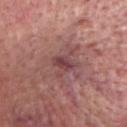<record>
<biopsy_status>not biopsied; imaged during a skin examination</biopsy_status>
<site>head or neck</site>
<lighting>white-light</lighting>
<patient>
  <sex>male</sex>
  <age_approx>80</age_approx>
</patient>
<lesion_size>
  <long_diameter_mm_approx>2.5</long_diameter_mm_approx>
</lesion_size>
<image>
  <source>total-body photography crop</source>
  <field_of_view_mm>15</field_of_view_mm>
</image>
</record>